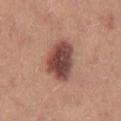Notes:
* notes: imaged on a skin check; not biopsied
* imaging modality: ~15 mm crop, total-body skin-cancer survey
* site: the right thigh
* lighting: white-light
* patient: female, about 25 years old
* lesion diameter: ≈5.5 mm